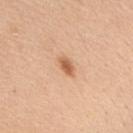biopsy status = total-body-photography surveillance lesion; no biopsy
subject = male, aged 58 to 62
size = ~2.5 mm (longest diameter)
anatomic site = the upper back
acquisition = ~15 mm crop, total-body skin-cancer survey